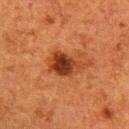Assessment: Recorded during total-body skin imaging; not selected for excision or biopsy. Context: A female patient, about 50 years old. This is a cross-polarized tile. This image is a 15 mm lesion crop taken from a total-body photograph. From the right upper arm.